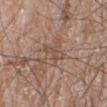Assessment: Imaged during a routine full-body skin examination; the lesion was not biopsied and no histopathology is available. Background: Automated image analysis of the tile measured a lesion area of about 3 mm² and a shape eccentricity near 0.95. The analysis additionally found an average lesion color of about L≈50 a*≈17 b*≈26 (CIELAB), roughly 6 lightness units darker than nearby skin, and a normalized lesion–skin contrast near 5. The analysis additionally found a classifier nevus-likeness of about 0/100 and a detector confidence of about 70 out of 100 that the crop contains a lesion. This is a white-light tile. The recorded lesion diameter is about 3.5 mm. A male subject, about 60 years old. The lesion is located on the right lower leg. A 15 mm close-up extracted from a 3D total-body photography capture.